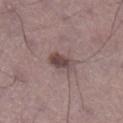<record>
  <biopsy_status>not biopsied; imaged during a skin examination</biopsy_status>
  <image>
    <source>total-body photography crop</source>
    <field_of_view_mm>15</field_of_view_mm>
  </image>
  <patient>
    <sex>male</sex>
    <age_approx>45</age_approx>
  </patient>
  <automated_metrics>
    <area_mm2_approx>5.0</area_mm2_approx>
    <shape_asymmetry>0.25</shape_asymmetry>
  </automated_metrics>
  <lesion_size>
    <long_diameter_mm_approx>3.0</long_diameter_mm_approx>
  </lesion_size>
  <site>right lower leg</site>
  <lighting>white-light</lighting>
</record>